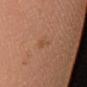Recorded during total-body skin imaging; not selected for excision or biopsy.
Automated tile analysis of the lesion measured an average lesion color of about L≈50 a*≈21 b*≈33 (CIELAB), roughly 5 lightness units darker than nearby skin, and a lesion-to-skin contrast of about 4.5 (normalized; higher = more distinct). The software also gave border irregularity of about 5 on a 0–10 scale, a color-variation rating of about 0/10, and radial color variation of about 0.
A lesion tile, about 15 mm wide, cut from a 3D total-body photograph.
The lesion is on the leg.
This is a cross-polarized tile.
The subject is a female about 70 years old.
The recorded lesion diameter is about 2.5 mm.